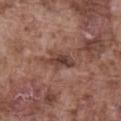follow-up: total-body-photography surveillance lesion; no biopsy
site: the front of the torso
patient: male, aged 73–77
size: ~4 mm (longest diameter)
image-analysis metrics: a mean CIELAB color near L≈41 a*≈20 b*≈24, about 10 CIELAB-L* units darker than the surrounding skin, and a normalized border contrast of about 8.5
imaging modality: total-body-photography crop, ~15 mm field of view
lighting: white-light